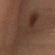Notes:
- workup: total-body-photography surveillance lesion; no biopsy
- tile lighting: cross-polarized
- lesion size: ≈11.5 mm
- patient: female, about 35 years old
- automated lesion analysis: an average lesion color of about L≈32 a*≈16 b*≈23 (CIELAB), a lesion–skin lightness drop of about 6, and a normalized lesion–skin contrast near 5.5
- site: the leg
- image source: 15 mm crop, total-body photography